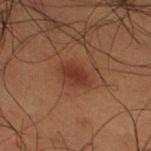This lesion was catalogued during total-body skin photography and was not selected for biopsy.
The lesion is located on the right thigh.
A male patient, aged around 50.
Automated image analysis of the tile measured a shape-asymmetry score of about 0.2 (0 = symmetric). And it measured a mean CIELAB color near L≈27 a*≈20 b*≈24 and about 7 CIELAB-L* units darker than the surrounding skin. And it measured a nevus-likeness score of about 65/100.
This is a cross-polarized tile.
Measured at roughly 3 mm in maximum diameter.
A close-up tile cropped from a whole-body skin photograph, about 15 mm across.